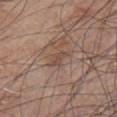Notes:
- workup: total-body-photography surveillance lesion; no biopsy
- TBP lesion metrics: a lesion area of about 3.5 mm², an outline eccentricity of about 0.85 (0 = round, 1 = elongated), and a symmetry-axis asymmetry near 0.35; a mean CIELAB color near L≈48 a*≈18 b*≈26, a lesion–skin lightness drop of about 7, and a lesion-to-skin contrast of about 5.5 (normalized; higher = more distinct); border irregularity of about 3.5 on a 0–10 scale, a color-variation rating of about 1/10, and peripheral color asymmetry of about 0.5; a lesion-detection confidence of about 90/100
- anatomic site: the front of the torso
- imaging modality: 15 mm crop, total-body photography
- lighting: white-light
- patient: male, aged approximately 70